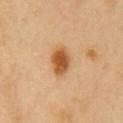{"biopsy_status": "not biopsied; imaged during a skin examination", "lighting": "cross-polarized", "automated_metrics": {"shape_asymmetry": 0.15, "border_irregularity_0_10": 1.5, "color_variation_0_10": 4.0, "peripheral_color_asymmetry": 1.0, "nevus_likeness_0_100": 100, "lesion_detection_confidence_0_100": 100}, "image": {"source": "total-body photography crop", "field_of_view_mm": 15}, "site": "chest", "patient": {"sex": "male", "age_approx": 50}}